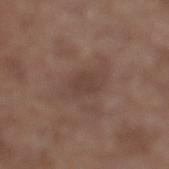notes = imaged on a skin check; not biopsied
lesion diameter = about 3 mm
imaging modality = ~15 mm tile from a whole-body skin photo
location = the left lower leg
illumination = white-light illumination
subject = male, roughly 65 years of age